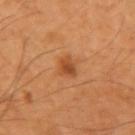Assessment:
No biopsy was performed on this lesion — it was imaged during a full skin examination and was not determined to be concerning.
Acquisition and patient details:
About 2.5 mm across. Cropped from a whole-body photographic skin survey; the tile spans about 15 mm. The lesion is located on the arm. The subject is a male in their mid- to late 50s. The tile uses cross-polarized illumination. Automated tile analysis of the lesion measured a mean CIELAB color near L≈44 a*≈24 b*≈37, a lesion–skin lightness drop of about 9, and a normalized border contrast of about 7. And it measured a border-irregularity index near 2.5/10, a color-variation rating of about 3.5/10, and radial color variation of about 1. The analysis additionally found a detector confidence of about 100 out of 100 that the crop contains a lesion.